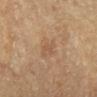Clinical impression: The lesion was tiled from a total-body skin photograph and was not biopsied. Clinical summary: This image is a 15 mm lesion crop taken from a total-body photograph. Captured under cross-polarized illumination. Longest diameter approximately 3 mm. From the right lower leg. A female subject, approximately 60 years of age.